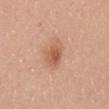Assessment:
Imaged during a routine full-body skin examination; the lesion was not biopsied and no histopathology is available.
Acquisition and patient details:
The patient is a female aged approximately 50. A roughly 15 mm field-of-view crop from a total-body skin photograph. Located on the mid back.